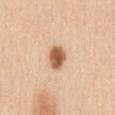Q: Where on the body is the lesion?
A: the chest
Q: What did automated image analysis measure?
A: an area of roughly 6 mm² and two-axis asymmetry of about 0.15
Q: Patient demographics?
A: female, approximately 30 years of age
Q: What lighting was used for the tile?
A: white-light illumination
Q: What kind of image is this?
A: ~15 mm crop, total-body skin-cancer survey